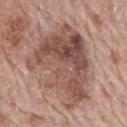{
  "biopsy_status": "not biopsied; imaged during a skin examination",
  "lesion_size": {
    "long_diameter_mm_approx": 9.0
  },
  "patient": {
    "sex": "male",
    "age_approx": 70
  },
  "automated_metrics": {
    "area_mm2_approx": 44.0,
    "eccentricity": 0.7,
    "shape_asymmetry": 0.4,
    "nevus_likeness_0_100": 0,
    "lesion_detection_confidence_0_100": 95
  },
  "lighting": "white-light",
  "site": "back",
  "image": {
    "source": "total-body photography crop",
    "field_of_view_mm": 15
  }
}